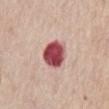Imaged during a routine full-body skin examination; the lesion was not biopsied and no histopathology is available. A close-up tile cropped from a whole-body skin photograph, about 15 mm across. On the front of the torso. A male subject in their mid- to late 70s. Approximately 4 mm at its widest. The tile uses white-light illumination.